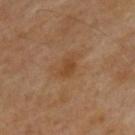Background: About 3 mm across. A male subject, aged 68–72. A close-up tile cropped from a whole-body skin photograph, about 15 mm across. The lesion is on the upper back. Automated image analysis of the tile measured a shape eccentricity near 0.75 and a shape-asymmetry score of about 0.2 (0 = symmetric). And it measured a border-irregularity index near 2/10, a color-variation rating of about 1.5/10, and radial color variation of about 0.5.